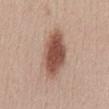| feature | finding |
|---|---|
| lesion diameter | ≈7 mm |
| lighting | white-light |
| image source | 15 mm crop, total-body photography |
| site | the abdomen |
| patient | female, aged approximately 25 |